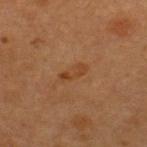diameter=≈3 mm | site=the upper back | subject=female, aged 38 to 42 | image source=~15 mm crop, total-body skin-cancer survey.